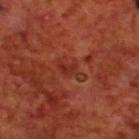The lesion was tiled from a total-body skin photograph and was not biopsied.